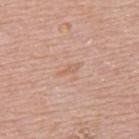<lesion>
  <biopsy_status>not biopsied; imaged during a skin examination</biopsy_status>
  <patient>
    <sex>male</sex>
    <age_approx>70</age_approx>
  </patient>
  <site>back</site>
  <lesion_size>
    <long_diameter_mm_approx>2.5</long_diameter_mm_approx>
  </lesion_size>
  <image>
    <source>total-body photography crop</source>
    <field_of_view_mm>15</field_of_view_mm>
  </image>
</lesion>